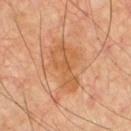Assessment:
Imaged during a routine full-body skin examination; the lesion was not biopsied and no histopathology is available.
Image and clinical context:
A male patient, aged around 60. On the chest. A roughly 15 mm field-of-view crop from a total-body skin photograph. An algorithmic analysis of the crop reported an average lesion color of about L≈57 a*≈24 b*≈39 (CIELAB), about 8 CIELAB-L* units darker than the surrounding skin, and a normalized border contrast of about 6.5. The analysis additionally found lesion-presence confidence of about 100/100. Captured under cross-polarized illumination. Measured at roughly 6 mm in maximum diameter.